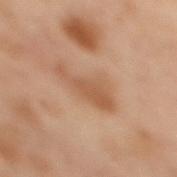{"biopsy_status": "not biopsied; imaged during a skin examination", "patient": {"sex": "female", "age_approx": 55}, "site": "back", "image": {"source": "total-body photography crop", "field_of_view_mm": 15}, "lesion_size": {"long_diameter_mm_approx": 5.5}, "lighting": "cross-polarized"}